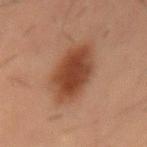{"biopsy_status": "not biopsied; imaged during a skin examination", "site": "abdomen", "lesion_size": {"long_diameter_mm_approx": 6.5}, "image": {"source": "total-body photography crop", "field_of_view_mm": 15}, "patient": {"sex": "male", "age_approx": 55}, "lighting": "cross-polarized"}